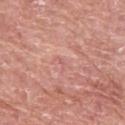No biopsy was performed on this lesion — it was imaged during a full skin examination and was not determined to be concerning.
A female subject, aged 58–62.
Automated tile analysis of the lesion measured a lesion area of about 1 mm², a shape eccentricity near 0.85, and two-axis asymmetry of about 0.4. It also reported an automated nevus-likeness rating near 0 out of 100.
A lesion tile, about 15 mm wide, cut from a 3D total-body photograph.
The lesion is located on the left upper arm.
Approximately 1 mm at its widest.
This is a white-light tile.